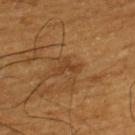Captured during whole-body skin photography for melanoma surveillance; the lesion was not biopsied. A male patient aged 58 to 62. The lesion is on the upper back. This is a cross-polarized tile. A 15 mm crop from a total-body photograph taken for skin-cancer surveillance. About 2 mm across.